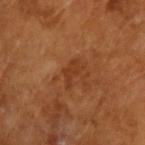Clinical impression:
Imaged during a routine full-body skin examination; the lesion was not biopsied and no histopathology is available.
Context:
The lesion's longest dimension is about 3 mm. A male subject aged around 65. The tile uses cross-polarized illumination. A 15 mm close-up tile from a total-body photography series done for melanoma screening.